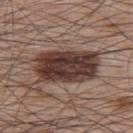Recorded during total-body skin imaging; not selected for excision or biopsy. Approximately 8 mm at its widest. A male patient roughly 65 years of age. From the upper back. A lesion tile, about 15 mm wide, cut from a 3D total-body photograph. This is a white-light tile. The total-body-photography lesion software estimated an outline eccentricity of about 0.85 (0 = round, 1 = elongated) and a symmetry-axis asymmetry near 0.2. The software also gave a classifier nevus-likeness of about 85/100 and lesion-presence confidence of about 100/100.